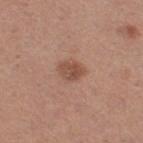– imaging modality — 15 mm crop, total-body photography
– site — the leg
– patient — female, about 30 years old
– automated metrics — a shape eccentricity near 0.65 and two-axis asymmetry of about 0.25; a mean CIELAB color near L≈49 a*≈22 b*≈28, roughly 10 lightness units darker than nearby skin, and a normalized lesion–skin contrast near 7.5; border irregularity of about 2 on a 0–10 scale, a color-variation rating of about 3.5/10, and radial color variation of about 1.5
– size — ~2.5 mm (longest diameter)
– tile lighting — white-light